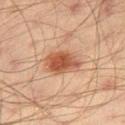biopsy status: no biopsy performed (imaged during a skin exam)
image source: total-body-photography crop, ~15 mm field of view
anatomic site: the right thigh
diameter: ~4.5 mm (longest diameter)
subject: male, aged 43 to 47